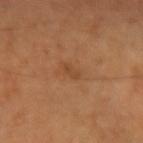Notes:
– TBP lesion metrics — a footprint of about 2.5 mm², an outline eccentricity of about 0.95 (0 = round, 1 = elongated), and a symmetry-axis asymmetry near 0.3; an automated nevus-likeness rating near 0 out of 100 and lesion-presence confidence of about 100/100
– subject — male, aged 53 to 57
– imaging modality — 15 mm crop, total-body photography
– anatomic site — the arm